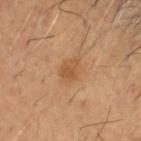notes: imaged on a skin check; not biopsied
imaging modality: ~15 mm tile from a whole-body skin photo
diameter: ≈2.5 mm
patient: male, aged around 60